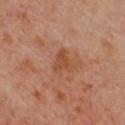Imaged during a routine full-body skin examination; the lesion was not biopsied and no histopathology is available. A female subject aged 58 to 62. The lesion is on the chest. A close-up tile cropped from a whole-body skin photograph, about 15 mm across.